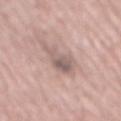| feature | finding |
|---|---|
| workup | catalogued during a skin exam; not biopsied |
| location | the mid back |
| lesion diameter | ~4.5 mm (longest diameter) |
| image source | ~15 mm crop, total-body skin-cancer survey |
| patient | male, roughly 70 years of age |
| tile lighting | white-light |